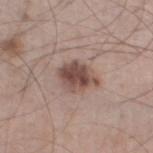The lesion was tiled from a total-body skin photograph and was not biopsied. A 15 mm close-up extracted from a 3D total-body photography capture. The lesion is on the left thigh. A male subject roughly 60 years of age.